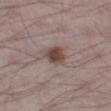This lesion was catalogued during total-body skin photography and was not selected for biopsy.
A lesion tile, about 15 mm wide, cut from a 3D total-body photograph.
The subject is a male approximately 70 years of age.
The lesion is located on the left thigh.